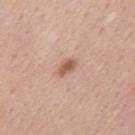biopsy_status: not biopsied; imaged during a skin examination
lesion_size:
  long_diameter_mm_approx: 2.5
patient:
  sex: male
  age_approx: 55
site: mid back
lighting: white-light
automated_metrics:
  area_mm2_approx: 3.5
  eccentricity: 0.8
  shape_asymmetry: 0.2
  cielab_L: 58
  cielab_a: 21
  cielab_b: 30
  vs_skin_contrast_norm: 7.5
image:
  source: total-body photography crop
  field_of_view_mm: 15